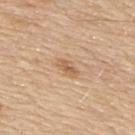Clinical impression: No biopsy was performed on this lesion — it was imaged during a full skin examination and was not determined to be concerning. Background: The lesion-visualizer software estimated a lesion area of about 4 mm², an eccentricity of roughly 0.85, and a shape-asymmetry score of about 0.3 (0 = symmetric). The software also gave a classifier nevus-likeness of about 35/100. A lesion tile, about 15 mm wide, cut from a 3D total-body photograph. The recorded lesion diameter is about 3 mm. A male subject in their 80s. Located on the upper back. This is a white-light tile.